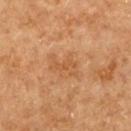This lesion was catalogued during total-body skin photography and was not selected for biopsy. The subject is a female aged approximately 60. A lesion tile, about 15 mm wide, cut from a 3D total-body photograph. From the upper back.